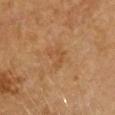<record>
  <biopsy_status>not biopsied; imaged during a skin examination</biopsy_status>
  <site>head or neck</site>
  <patient>
    <sex>female</sex>
    <age_approx>65</age_approx>
  </patient>
  <image>
    <source>total-body photography crop</source>
    <field_of_view_mm>15</field_of_view_mm>
  </image>
</record>